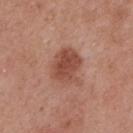Assessment:
Imaged during a routine full-body skin examination; the lesion was not biopsied and no histopathology is available.
Image and clinical context:
A close-up tile cropped from a whole-body skin photograph, about 15 mm across. The lesion is on the upper back. The lesion's longest dimension is about 4 mm. A female patient, roughly 40 years of age. Imaged with white-light lighting.